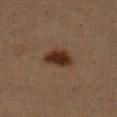| feature | finding |
|---|---|
| anatomic site | the arm |
| patient | male, in their 50s |
| image source | ~15 mm crop, total-body skin-cancer survey |